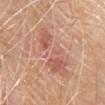follow-up: total-body-photography surveillance lesion; no biopsy
location: the chest
patient: female, approximately 70 years of age
image: 15 mm crop, total-body photography
TBP lesion metrics: a lesion area of about 23 mm² and an eccentricity of roughly 0.95; border irregularity of about 8 on a 0–10 scale, a within-lesion color-variation index near 5.5/10, and a peripheral color-asymmetry measure near 1.5; a detector confidence of about 100 out of 100 that the crop contains a lesion
tile lighting: white-light
diameter: about 9 mm